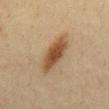| field | value |
|---|---|
| size | about 5.5 mm |
| imaging modality | ~15 mm crop, total-body skin-cancer survey |
| image-analysis metrics | a mean CIELAB color near L≈46 a*≈17 b*≈32, a lesion–skin lightness drop of about 12, and a lesion-to-skin contrast of about 9 (normalized; higher = more distinct) |
| illumination | cross-polarized illumination |
| patient | male, in their mid- to late 30s |
| anatomic site | the mid back |